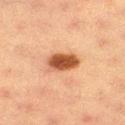Assessment:
Part of a total-body skin-imaging series; this lesion was reviewed on a skin check and was not flagged for biopsy.
Acquisition and patient details:
A female subject, approximately 55 years of age. A 15 mm close-up tile from a total-body photography series done for melanoma screening. The lesion is on the right thigh.